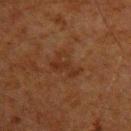Impression:
The lesion was photographed on a routine skin check and not biopsied; there is no pathology result.
Context:
A male subject, approximately 60 years of age. A region of skin cropped from a whole-body photographic capture, roughly 15 mm wide. About 3.5 mm across. The lesion is on the left upper arm.